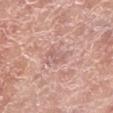The lesion was photographed on a routine skin check and not biopsied; there is no pathology result. The lesion is on the left lower leg. Measured at roughly 3 mm in maximum diameter. A 15 mm crop from a total-body photograph taken for skin-cancer surveillance. A male subject, in their mid-50s. Automated image analysis of the tile measured a footprint of about 3.5 mm² and an eccentricity of roughly 0.9. The analysis additionally found roughly 7 lightness units darker than nearby skin and a normalized lesion–skin contrast near 4.5.